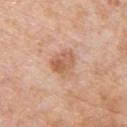Case summary:
- biopsy status: no biopsy performed (imaged during a skin exam)
- subject: male, about 60 years old
- image: ~15 mm crop, total-body skin-cancer survey
- anatomic site: the upper back
- lighting: white-light illumination
- diameter: ≈3.5 mm